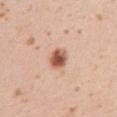Clinical summary: The lesion-visualizer software estimated a footprint of about 5.5 mm², a shape eccentricity near 0.7, and a shape-asymmetry score of about 0.1 (0 = symmetric). The analysis additionally found a border-irregularity rating of about 1/10, a within-lesion color-variation index near 6/10, and radial color variation of about 1.5. And it measured a detector confidence of about 100 out of 100 that the crop contains a lesion. A 15 mm crop from a total-body photograph taken for skin-cancer surveillance. A female patient roughly 20 years of age. Measured at roughly 3 mm in maximum diameter. Captured under white-light illumination. Located on the right upper arm.